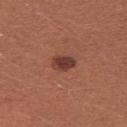workup: catalogued during a skin exam; not biopsied | patient: female, aged around 25 | image: total-body-photography crop, ~15 mm field of view | diameter: ≈3 mm | anatomic site: the right upper arm.